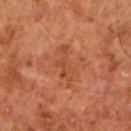Q: Was this lesion biopsied?
A: imaged on a skin check; not biopsied